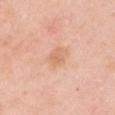* anatomic site: the chest
* illumination: white-light illumination
* lesion size: about 3 mm
* TBP lesion metrics: a lesion color around L≈68 a*≈22 b*≈35 in CIELAB, about 7 CIELAB-L* units darker than the surrounding skin, and a normalized lesion–skin contrast near 5.5; a border-irregularity index near 2/10, internal color variation of about 2.5 on a 0–10 scale, and radial color variation of about 1
* subject: female, approximately 50 years of age
* acquisition: 15 mm crop, total-body photography